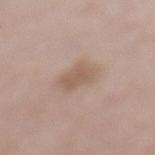Q: Was a biopsy performed?
A: no biopsy performed (imaged during a skin exam)
Q: What is the imaging modality?
A: total-body-photography crop, ~15 mm field of view
Q: What are the patient's age and sex?
A: female, about 60 years old
Q: What did automated image analysis measure?
A: a footprint of about 7 mm², an outline eccentricity of about 0.55 (0 = round, 1 = elongated), and a shape-asymmetry score of about 0.15 (0 = symmetric); an automated nevus-likeness rating near 10 out of 100 and lesion-presence confidence of about 100/100
Q: Lesion location?
A: the left lower leg
Q: How large is the lesion?
A: ~3 mm (longest diameter)